Located on the abdomen · the subject is a female aged approximately 55 · the recorded lesion diameter is about 7.5 mm · a 15 mm crop from a total-body photograph taken for skin-cancer surveillance · Automated image analysis of the tile measured a lesion area of about 28 mm², an outline eccentricity of about 0.75 (0 = round, 1 = elongated), and a shape-asymmetry score of about 0.1 (0 = symmetric). The analysis additionally found border irregularity of about 1.5 on a 0–10 scale, a color-variation rating of about 9.5/10, and peripheral color asymmetry of about 3. The analysis additionally found a classifier nevus-likeness of about 25/100:
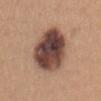The biopsy diagnosis was a borderline lesion of uncertain malignant potential: atypical melanocytic neoplasm.